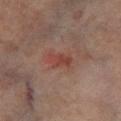No biopsy was performed on this lesion — it was imaged during a full skin examination and was not determined to be concerning. A male patient approximately 70 years of age. A 15 mm close-up extracted from a 3D total-body photography capture. The recorded lesion diameter is about 3 mm. Located on the right lower leg.